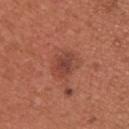Assessment:
No biopsy was performed on this lesion — it was imaged during a full skin examination and was not determined to be concerning.
Clinical summary:
The lesion is located on the upper back. The patient is a female approximately 30 years of age. A region of skin cropped from a whole-body photographic capture, roughly 15 mm wide. Longest diameter approximately 3 mm. The total-body-photography lesion software estimated a lesion color around L≈43 a*≈27 b*≈28 in CIELAB and roughly 9 lightness units darker than nearby skin. The analysis additionally found a border-irregularity rating of about 3/10 and a within-lesion color-variation index near 3/10. The software also gave an automated nevus-likeness rating near 90 out of 100 and a lesion-detection confidence of about 100/100. Imaged with white-light lighting.